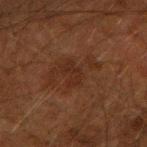No biopsy was performed on this lesion — it was imaged during a full skin examination and was not determined to be concerning. Located on the right upper arm. A male patient, aged around 50. A 15 mm crop from a total-body photograph taken for skin-cancer surveillance. Imaged with cross-polarized lighting. An algorithmic analysis of the crop reported a lesion area of about 3 mm², an outline eccentricity of about 0.85 (0 = round, 1 = elongated), and a shape-asymmetry score of about 0.45 (0 = symmetric). The analysis additionally found about 4 CIELAB-L* units darker than the surrounding skin and a normalized lesion–skin contrast near 5.5. The software also gave a classifier nevus-likeness of about 0/100 and a lesion-detection confidence of about 100/100.